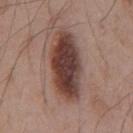A lesion tile, about 15 mm wide, cut from a 3D total-body photograph. The lesion is on the front of the torso. A male patient roughly 55 years of age. The tile uses white-light illumination.